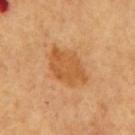Clinical impression:
The lesion was tiled from a total-body skin photograph and was not biopsied.
Acquisition and patient details:
The lesion is located on the mid back. Longest diameter approximately 5.5 mm. A male subject aged 63–67. A 15 mm close-up extracted from a 3D total-body photography capture. This is a cross-polarized tile.